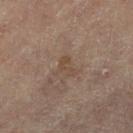| feature | finding |
|---|---|
| workup | catalogued during a skin exam; not biopsied |
| automated lesion analysis | a shape-asymmetry score of about 0.6 (0 = symmetric); an automated nevus-likeness rating near 0 out of 100 |
| patient | female, aged 78–82 |
| acquisition | ~15 mm crop, total-body skin-cancer survey |
| body site | the left leg |
| illumination | cross-polarized |
| size | ≈2.5 mm |